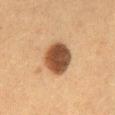Assessment:
The lesion was tiled from a total-body skin photograph and was not biopsied.
Background:
Located on the front of the torso. A close-up tile cropped from a whole-body skin photograph, about 15 mm across. Imaged with cross-polarized lighting. A female subject aged 48 to 52.